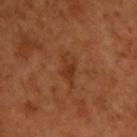Recorded during total-body skin imaging; not selected for excision or biopsy.
The lesion is located on the upper back.
A region of skin cropped from a whole-body photographic capture, roughly 15 mm wide.
A male patient, aged approximately 50.
Imaged with cross-polarized lighting.